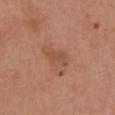Captured during whole-body skin photography for melanoma surveillance; the lesion was not biopsied.
On the chest.
The lesion-visualizer software estimated a shape eccentricity near 0.9 and a shape-asymmetry score of about 0.3 (0 = symmetric). It also reported an average lesion color of about L≈50 a*≈23 b*≈30 (CIELAB) and roughly 7 lightness units darker than nearby skin. And it measured a nevus-likeness score of about 15/100 and lesion-presence confidence of about 100/100.
The patient is a female aged approximately 65.
Measured at roughly 3.5 mm in maximum diameter.
A close-up tile cropped from a whole-body skin photograph, about 15 mm across.
Captured under white-light illumination.